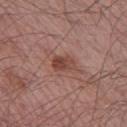Impression:
The lesion was photographed on a routine skin check and not biopsied; there is no pathology result.
Clinical summary:
Cropped from a whole-body photographic skin survey; the tile spans about 15 mm. The lesion is located on the left thigh. A male subject in their mid-50s.